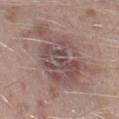| field | value |
|---|---|
| workup | imaged on a skin check; not biopsied |